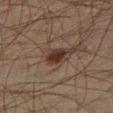A region of skin cropped from a whole-body photographic capture, roughly 15 mm wide. This is a cross-polarized tile. Measured at roughly 2.5 mm in maximum diameter. Automated tile analysis of the lesion measured a mean CIELAB color near L≈24 a*≈14 b*≈20 and about 9 CIELAB-L* units darker than the surrounding skin. And it measured a peripheral color-asymmetry measure near 0.5. The subject is a male aged 48 to 52. From the leg.